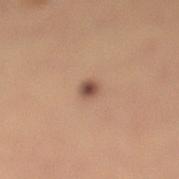Case summary:
– follow-up: catalogued during a skin exam; not biopsied
– patient: female, roughly 35 years of age
– image-analysis metrics: a shape eccentricity near 0.65 and a shape-asymmetry score of about 0.25 (0 = symmetric); a border-irregularity index near 2/10, a color-variation rating of about 2.5/10, and radial color variation of about 1; an automated nevus-likeness rating near 95 out of 100 and a lesion-detection confidence of about 100/100
– image: ~15 mm crop, total-body skin-cancer survey
– body site: the left lower leg
– lighting: cross-polarized
– diameter: about 2 mm